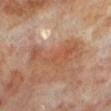<record>
<biopsy_status>not biopsied; imaged during a skin examination</biopsy_status>
<site>left lower leg</site>
<lighting>cross-polarized</lighting>
<automated_metrics>
  <area_mm2_approx>11.0</area_mm2_approx>
  <eccentricity>0.9</eccentricity>
  <shape_asymmetry>0.7</shape_asymmetry>
</automated_metrics>
<patient>
  <sex>male</sex>
  <age_approx>70</age_approx>
</patient>
<image>
  <source>total-body photography crop</source>
  <field_of_view_mm>15</field_of_view_mm>
</image>
</record>